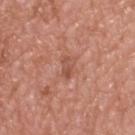{
  "biopsy_status": "not biopsied; imaged during a skin examination",
  "lighting": "white-light",
  "site": "upper back",
  "patient": {
    "sex": "male",
    "age_approx": 50
  },
  "image": {
    "source": "total-body photography crop",
    "field_of_view_mm": 15
  },
  "automated_metrics": {
    "vs_skin_darker_L": 8.0,
    "vs_skin_contrast_norm": 5.5
  },
  "lesion_size": {
    "long_diameter_mm_approx": 3.0
  }
}